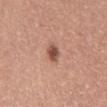{
  "biopsy_status": "not biopsied; imaged during a skin examination",
  "lighting": "white-light",
  "site": "abdomen",
  "patient": {
    "sex": "female",
    "age_approx": 50
  },
  "image": {
    "source": "total-body photography crop",
    "field_of_view_mm": 15
  },
  "lesion_size": {
    "long_diameter_mm_approx": 2.5
  }
}